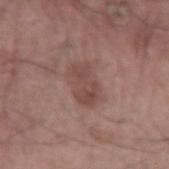<record>
<biopsy_status>not biopsied; imaged during a skin examination</biopsy_status>
<automated_metrics>
  <nevus_likeness_0_100>5</nevus_likeness_0_100>
  <lesion_detection_confidence_0_100>100</lesion_detection_confidence_0_100>
</automated_metrics>
<site>right upper arm</site>
<lesion_size>
  <long_diameter_mm_approx>4.5</long_diameter_mm_approx>
</lesion_size>
<image>
  <source>total-body photography crop</source>
  <field_of_view_mm>15</field_of_view_mm>
</image>
<lighting>white-light</lighting>
<patient>
  <sex>male</sex>
  <age_approx>65</age_approx>
</patient>
</record>